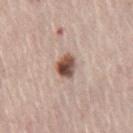workup: catalogued during a skin exam; not biopsied
illumination: white-light
subject: male, in their mid-60s
imaging modality: 15 mm crop, total-body photography
size: ≈3 mm
body site: the leg
TBP lesion metrics: a lesion area of about 6 mm², an eccentricity of roughly 0.55, and a symmetry-axis asymmetry near 0.2; an average lesion color of about L≈51 a*≈19 b*≈26 (CIELAB) and about 18 CIELAB-L* units darker than the surrounding skin; a border-irregularity index near 2/10, a within-lesion color-variation index near 8.5/10, and radial color variation of about 2.5; a nevus-likeness score of about 95/100 and a lesion-detection confidence of about 100/100